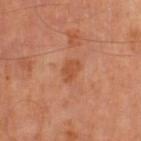<tbp_lesion>
<biopsy_status>not biopsied; imaged during a skin examination</biopsy_status>
<patient>
  <sex>male</sex>
  <age_approx>65</age_approx>
</patient>
<site>left thigh</site>
<image>
  <source>total-body photography crop</source>
  <field_of_view_mm>15</field_of_view_mm>
</image>
</tbp_lesion>